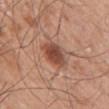patient = male, aged approximately 55 | location = the right upper arm | TBP lesion metrics = an area of roughly 8.5 mm²; an average lesion color of about L≈48 a*≈23 b*≈29 (CIELAB) and a normalized border contrast of about 9; a color-variation rating of about 4.5/10 and a peripheral color-asymmetry measure near 1.5 | lesion size = ~4 mm (longest diameter) | image = ~15 mm crop, total-body skin-cancer survey | lighting = white-light.